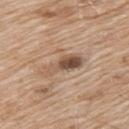Impression: Part of a total-body skin-imaging series; this lesion was reviewed on a skin check and was not flagged for biopsy. Acquisition and patient details: A lesion tile, about 15 mm wide, cut from a 3D total-body photograph. Automated tile analysis of the lesion measured a border-irregularity rating of about 4.5/10, a color-variation rating of about 8/10, and peripheral color asymmetry of about 3. It also reported lesion-presence confidence of about 100/100. The recorded lesion diameter is about 5.5 mm. On the upper back. This is a white-light tile. A male subject, roughly 65 years of age.